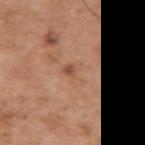Recorded during total-body skin imaging; not selected for excision or biopsy. Automated image analysis of the tile measured an area of roughly 4.5 mm² and a symmetry-axis asymmetry near 0.6. And it measured a border-irregularity rating of about 6/10, internal color variation of about 3 on a 0–10 scale, and radial color variation of about 1. The analysis additionally found a classifier nevus-likeness of about 0/100 and lesion-presence confidence of about 100/100. Captured under white-light illumination. The subject is a male in their mid- to late 50s. Located on the right upper arm. A 15 mm close-up extracted from a 3D total-body photography capture. Measured at roughly 3 mm in maximum diameter.